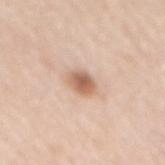Q: Is there a histopathology result?
A: imaged on a skin check; not biopsied